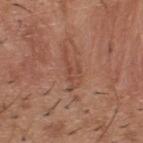This lesion was catalogued during total-body skin photography and was not selected for biopsy. The patient is a male aged approximately 40. Cropped from a whole-body photographic skin survey; the tile spans about 15 mm. On the upper back. About 5 mm across.